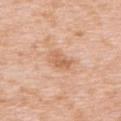{
  "biopsy_status": "not biopsied; imaged during a skin examination",
  "lighting": "white-light",
  "image": {
    "source": "total-body photography crop",
    "field_of_view_mm": 15
  },
  "patient": {
    "sex": "female",
    "age_approx": 40
  },
  "lesion_size": {
    "long_diameter_mm_approx": 3.0
  },
  "site": "upper back"
}